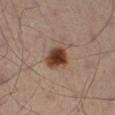The lesion is located on the left leg. Cropped from a total-body skin-imaging series; the visible field is about 15 mm. Measured at roughly 3.5 mm in maximum diameter. An algorithmic analysis of the crop reported an area of roughly 7.5 mm², a shape eccentricity near 0.5, and a shape-asymmetry score of about 0.2 (0 = symmetric). The software also gave a lesion–skin lightness drop of about 15 and a normalized lesion–skin contrast near 12.5. And it measured border irregularity of about 2 on a 0–10 scale and a peripheral color-asymmetry measure near 1.5. And it measured a nevus-likeness score of about 100/100. A male subject, aged around 50. Imaged with cross-polarized lighting.